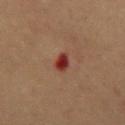<tbp_lesion>
<biopsy_status>not biopsied; imaged during a skin examination</biopsy_status>
<site>mid back</site>
<patient>
  <sex>female</sex>
  <age_approx>80</age_approx>
</patient>
<lesion_size>
  <long_diameter_mm_approx>2.5</long_diameter_mm_approx>
</lesion_size>
<image>
  <source>total-body photography crop</source>
  <field_of_view_mm>15</field_of_view_mm>
</image>
<lighting>cross-polarized</lighting>
<automated_metrics>
  <cielab_L>26</cielab_L>
  <cielab_a>23</cielab_a>
  <cielab_b>22</cielab_b>
  <vs_skin_darker_L>10.0</vs_skin_darker_L>
  <vs_skin_contrast_norm>10.5</vs_skin_contrast_norm>
</automated_metrics>
</tbp_lesion>